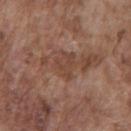Clinical impression: Imaged during a routine full-body skin examination; the lesion was not biopsied and no histopathology is available. Context: Imaged with white-light lighting. The lesion is on the chest. A male patient aged approximately 75. A close-up tile cropped from a whole-body skin photograph, about 15 mm across. The total-body-photography lesion software estimated a footprint of about 6.5 mm², an outline eccentricity of about 0.6 (0 = round, 1 = elongated), and a symmetry-axis asymmetry near 0.35. The analysis additionally found roughly 7 lightness units darker than nearby skin and a normalized lesion–skin contrast near 5.5. And it measured a color-variation rating of about 2.5/10 and a peripheral color-asymmetry measure near 1. It also reported a nevus-likeness score of about 0/100. The lesion's longest dimension is about 3.5 mm.